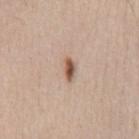workup = imaged on a skin check; not biopsied
tile lighting = white-light
patient = male, aged approximately 50
body site = the chest
size = ~3 mm (longest diameter)
image source = total-body-photography crop, ~15 mm field of view